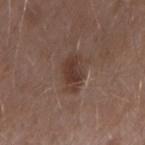Q: Was this lesion biopsied?
A: catalogued during a skin exam; not biopsied
Q: How was the tile lit?
A: white-light
Q: What kind of image is this?
A: ~15 mm crop, total-body skin-cancer survey
Q: What is the anatomic site?
A: the right upper arm
Q: Patient demographics?
A: male, approximately 55 years of age
Q: What is the lesion's diameter?
A: ~4 mm (longest diameter)
Q: What did automated image analysis measure?
A: an average lesion color of about L≈37 a*≈17 b*≈23 (CIELAB) and a lesion–skin lightness drop of about 9